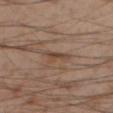Findings:
- workup — total-body-photography surveillance lesion; no biopsy
- illumination — cross-polarized illumination
- anatomic site — the right thigh
- imaging modality — total-body-photography crop, ~15 mm field of view
- patient — male, roughly 55 years of age